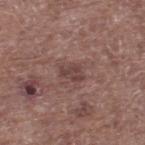  biopsy_status: not biopsied; imaged during a skin examination
  lighting: white-light
  site: right lower leg
  lesion_size:
    long_diameter_mm_approx: 2.5
  patient:
    sex: male
    age_approx: 70
  image:
    source: total-body photography crop
    field_of_view_mm: 15